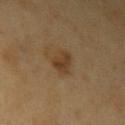The lesion was photographed on a routine skin check and not biopsied; there is no pathology result. A 15 mm close-up tile from a total-body photography series done for melanoma screening. A male patient, aged 58 to 62. The total-body-photography lesion software estimated a border-irregularity index near 2.5/10, a within-lesion color-variation index near 3/10, and a peripheral color-asymmetry measure near 1. The analysis additionally found a classifier nevus-likeness of about 75/100 and a detector confidence of about 100 out of 100 that the crop contains a lesion. This is a cross-polarized tile. Approximately 3 mm at its widest. From the left upper arm.